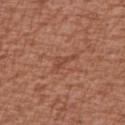This lesion was catalogued during total-body skin photography and was not selected for biopsy.
Longest diameter approximately 3.5 mm.
A female subject approximately 75 years of age.
Located on the arm.
A close-up tile cropped from a whole-body skin photograph, about 15 mm across.